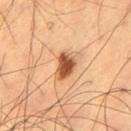• workup — total-body-photography surveillance lesion; no biopsy
• subject — male, approximately 60 years of age
• image — ~15 mm tile from a whole-body skin photo
• anatomic site — the leg